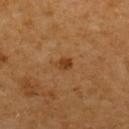Clinical impression: The lesion was photographed on a routine skin check and not biopsied; there is no pathology result. Context: A lesion tile, about 15 mm wide, cut from a 3D total-body photograph. A female subject, aged approximately 55. The lesion is on the upper back. The lesion's longest dimension is about 2 mm.